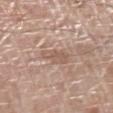<case>
<image>
  <source>total-body photography crop</source>
  <field_of_view_mm>15</field_of_view_mm>
</image>
<patient>
  <sex>male</sex>
  <age_approx>70</age_approx>
</patient>
<site>left lower leg</site>
<lesion_size>
  <long_diameter_mm_approx>3.5</long_diameter_mm_approx>
</lesion_size>
<lighting>white-light</lighting>
</case>